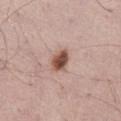Captured during whole-body skin photography for melanoma surveillance; the lesion was not biopsied.